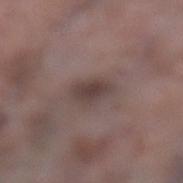Clinical impression: The lesion was photographed on a routine skin check and not biopsied; there is no pathology result. Acquisition and patient details: From the leg. The patient is a female roughly 70 years of age. A close-up tile cropped from a whole-body skin photograph, about 15 mm across.